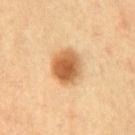biopsy status: total-body-photography surveillance lesion; no biopsy
anatomic site: the chest
subject: male, in their 70s
image: ~15 mm crop, total-body skin-cancer survey
image-analysis metrics: a lesion color around L≈59 a*≈22 b*≈40 in CIELAB, roughly 15 lightness units darker than nearby skin, and a lesion-to-skin contrast of about 9.5 (normalized; higher = more distinct); border irregularity of about 1 on a 0–10 scale and a within-lesion color-variation index near 6/10; a nevus-likeness score of about 100/100 and a detector confidence of about 100 out of 100 that the crop contains a lesion
lesion size: ≈4 mm